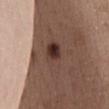This lesion was catalogued during total-body skin photography and was not selected for biopsy.
This image is a 15 mm lesion crop taken from a total-body photograph.
Longest diameter approximately 5.5 mm.
The total-body-photography lesion software estimated a lesion color around L≈38 a*≈17 b*≈22 in CIELAB, roughly 11 lightness units darker than nearby skin, and a normalized border contrast of about 9.
From the chest.
A female patient, in their 40s.
Imaged with white-light lighting.